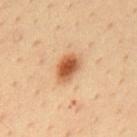Imaged during a routine full-body skin examination; the lesion was not biopsied and no histopathology is available.
Captured under cross-polarized illumination.
Automated image analysis of the tile measured a lesion area of about 7 mm² and an eccentricity of roughly 0.75. The analysis additionally found a lesion color around L≈53 a*≈23 b*≈36 in CIELAB and about 15 CIELAB-L* units darker than the surrounding skin.
A lesion tile, about 15 mm wide, cut from a 3D total-body photograph.
Longest diameter approximately 3.5 mm.
On the mid back.
A male subject, in their mid- to late 30s.